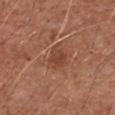Q: Was this lesion biopsied?
A: total-body-photography surveillance lesion; no biopsy
Q: Automated lesion metrics?
A: border irregularity of about 3 on a 0–10 scale, a color-variation rating of about 3/10, and a peripheral color-asymmetry measure near 1; a classifier nevus-likeness of about 25/100
Q: What is the imaging modality?
A: ~15 mm crop, total-body skin-cancer survey
Q: What are the patient's age and sex?
A: male, aged 63 to 67
Q: Where on the body is the lesion?
A: the left forearm
Q: What lighting was used for the tile?
A: white-light